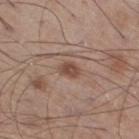Findings:
• biopsy status — imaged on a skin check; not biopsied
• lighting — white-light
• imaging modality — ~15 mm crop, total-body skin-cancer survey
• image-analysis metrics — an area of roughly 3.5 mm², an outline eccentricity of about 0.7 (0 = round, 1 = elongated), and two-axis asymmetry of about 0.25; border irregularity of about 2 on a 0–10 scale, a color-variation rating of about 2.5/10, and a peripheral color-asymmetry measure near 1
• body site — the right thigh
• size — ~2.5 mm (longest diameter)
• patient — male, aged 58 to 62